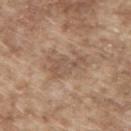| feature | finding |
|---|---|
| lighting | white-light |
| patient | male, aged 63–67 |
| diameter | ≈4.5 mm |
| body site | the upper back |
| acquisition | ~15 mm crop, total-body skin-cancer survey |
| image-analysis metrics | an area of roughly 8 mm² and an outline eccentricity of about 0.85 (0 = round, 1 = elongated); an average lesion color of about L≈53 a*≈16 b*≈28 (CIELAB), a lesion–skin lightness drop of about 8, and a normalized border contrast of about 5.5; a border-irregularity index near 6.5/10 and a peripheral color-asymmetry measure near 0.5; a detector confidence of about 95 out of 100 that the crop contains a lesion |